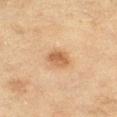Imaged with cross-polarized lighting. Automated image analysis of the tile measured a classifier nevus-likeness of about 90/100 and lesion-presence confidence of about 100/100. Cropped from a whole-body photographic skin survey; the tile spans about 15 mm. A female patient, roughly 55 years of age. From the left thigh.